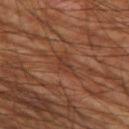follow-up: imaged on a skin check; not biopsied | imaging modality: 15 mm crop, total-body photography | anatomic site: the left thigh | automated metrics: an area of roughly 3 mm², an outline eccentricity of about 0.8 (0 = round, 1 = elongated), and a symmetry-axis asymmetry near 0.35; an average lesion color of about L≈36 a*≈22 b*≈28 (CIELAB), about 6 CIELAB-L* units darker than the surrounding skin, and a normalized lesion–skin contrast near 5.5 | subject: male, approximately 60 years of age.